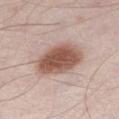{"biopsy_status": "not biopsied; imaged during a skin examination", "image": {"source": "total-body photography crop", "field_of_view_mm": 15}, "patient": {"sex": "male", "age_approx": 55}, "lesion_size": {"long_diameter_mm_approx": 5.5}, "automated_metrics": {"color_variation_0_10": 4.0, "nevus_likeness_0_100": 100, "lesion_detection_confidence_0_100": 100}, "site": "right thigh"}